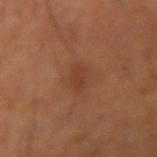workup=imaged on a skin check; not biopsied
imaging modality=~15 mm tile from a whole-body skin photo
location=the right forearm
subject=male, approximately 50 years of age
lesion size=about 3 mm
tile lighting=cross-polarized